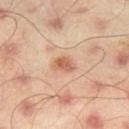This lesion was catalogued during total-body skin photography and was not selected for biopsy. This is a cross-polarized tile. Automated image analysis of the tile measured a border-irregularity rating of about 2/10. The lesion's longest dimension is about 2.5 mm. The subject is a male approximately 45 years of age. A close-up tile cropped from a whole-body skin photograph, about 15 mm across. The lesion is on the right thigh.